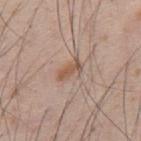notes: catalogued during a skin exam; not biopsied
automated lesion analysis: border irregularity of about 4.5 on a 0–10 scale, internal color variation of about 0.5 on a 0–10 scale, and radial color variation of about 0; an automated nevus-likeness rating near 55 out of 100 and lesion-presence confidence of about 100/100
tile lighting: white-light
subject: male, aged approximately 35
location: the upper back
image source: total-body-photography crop, ~15 mm field of view